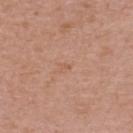No biopsy was performed on this lesion — it was imaged during a full skin examination and was not determined to be concerning. A lesion tile, about 15 mm wide, cut from a 3D total-body photograph. This is a white-light tile. The lesion is located on the head or neck. A male patient, approximately 55 years of age. The recorded lesion diameter is about 1 mm.